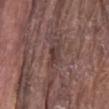The lesion is on the abdomen. This is a white-light tile. A 15 mm close-up extracted from a 3D total-body photography capture. A male subject approximately 80 years of age. Measured at roughly 3 mm in maximum diameter.